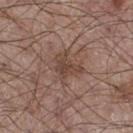Q: Was this lesion biopsied?
A: total-body-photography surveillance lesion; no biopsy
Q: How large is the lesion?
A: ~3.5 mm (longest diameter)
Q: What are the patient's age and sex?
A: male, aged 58–62
Q: Lesion location?
A: the right thigh
Q: Automated lesion metrics?
A: a mean CIELAB color near L≈43 a*≈18 b*≈24, about 8 CIELAB-L* units darker than the surrounding skin, and a normalized border contrast of about 7; an automated nevus-likeness rating near 0 out of 100 and a lesion-detection confidence of about 100/100
Q: What is the imaging modality?
A: ~15 mm tile from a whole-body skin photo
Q: How was the tile lit?
A: white-light illumination